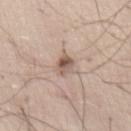| feature | finding |
|---|---|
| workup | no biopsy performed (imaged during a skin exam) |
| image source | ~15 mm tile from a whole-body skin photo |
| body site | the front of the torso |
| image-analysis metrics | an area of roughly 4 mm², an outline eccentricity of about 0.65 (0 = round, 1 = elongated), and two-axis asymmetry of about 0.25; a mean CIELAB color near L≈56 a*≈16 b*≈25, a lesion–skin lightness drop of about 12, and a normalized border contrast of about 8 |
| diameter | about 2.5 mm |
| subject | male, roughly 65 years of age |